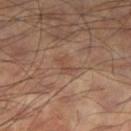Captured during whole-body skin photography for melanoma surveillance; the lesion was not biopsied. The patient is a male in their 60s. This is a cross-polarized tile. The lesion is located on the right thigh. Cropped from a whole-body photographic skin survey; the tile spans about 15 mm. Longest diameter approximately 2.5 mm.